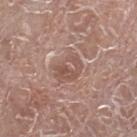{
  "biopsy_status": "not biopsied; imaged during a skin examination",
  "image": {
    "source": "total-body photography crop",
    "field_of_view_mm": 15
  },
  "site": "leg",
  "patient": {
    "sex": "male",
    "age_approx": 75
  }
}